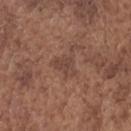follow-up — catalogued during a skin exam; not biopsied
anatomic site — the head or neck
patient — male, in their mid-70s
lighting — white-light
automated lesion analysis — a lesion area of about 5 mm² and a shape eccentricity near 0.65; a color-variation rating of about 1.5/10 and radial color variation of about 0.5; an automated nevus-likeness rating near 10 out of 100
image source — 15 mm crop, total-body photography
lesion size — ~3 mm (longest diameter)